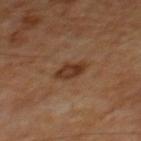Clinical impression:
Imaged during a routine full-body skin examination; the lesion was not biopsied and no histopathology is available.
Acquisition and patient details:
The patient is a male aged around 65. Captured under cross-polarized illumination. A region of skin cropped from a whole-body photographic capture, roughly 15 mm wide. Located on the mid back. About 3.5 mm across.